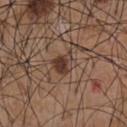Q: Was this lesion biopsied?
A: catalogued during a skin exam; not biopsied
Q: How was this image acquired?
A: ~15 mm crop, total-body skin-cancer survey
Q: What is the anatomic site?
A: the front of the torso
Q: What are the patient's age and sex?
A: male, aged 53–57